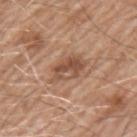biopsy status: total-body-photography surveillance lesion; no biopsy
body site: the left upper arm
patient: male, aged around 60
image: ~15 mm crop, total-body skin-cancer survey
size: ≈4 mm